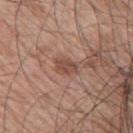This lesion was catalogued during total-body skin photography and was not selected for biopsy. Cropped from a total-body skin-imaging series; the visible field is about 15 mm. From the left arm. A male subject aged around 70. This is a white-light tile.